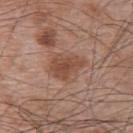workup: total-body-photography surveillance lesion; no biopsy | size: about 4.5 mm | illumination: white-light | image: 15 mm crop, total-body photography | subject: male, aged 63 to 67 | TBP lesion metrics: an average lesion color of about L≈48 a*≈21 b*≈28 (CIELAB), a lesion–skin lightness drop of about 8, and a normalized lesion–skin contrast near 7 | anatomic site: the upper back.